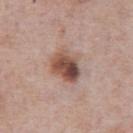Part of a total-body skin-imaging series; this lesion was reviewed on a skin check and was not flagged for biopsy.
The lesion is on the chest.
A close-up tile cropped from a whole-body skin photograph, about 15 mm across.
A male subject, roughly 75 years of age.
The tile uses white-light illumination.